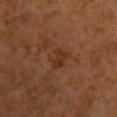Notes:
* notes: no biopsy performed (imaged during a skin exam)
* acquisition: ~15 mm crop, total-body skin-cancer survey
* anatomic site: the right upper arm
* automated lesion analysis: an area of roughly 4 mm² and a shape eccentricity near 0.75; a classifier nevus-likeness of about 5/100
* patient: female, approximately 55 years of age